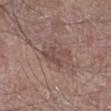follow-up: total-body-photography surveillance lesion; no biopsy | imaging modality: ~15 mm tile from a whole-body skin photo | location: the leg | patient: male, approximately 60 years of age | tile lighting: white-light illumination | diameter: ~3 mm (longest diameter).